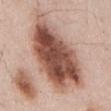Q: Is there a histopathology result?
A: imaged on a skin check; not biopsied